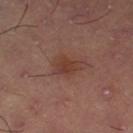Impression: This lesion was catalogued during total-body skin photography and was not selected for biopsy. Context: A 15 mm close-up extracted from a 3D total-body photography capture. From the left thigh.